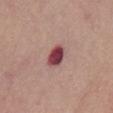Assessment: The lesion was photographed on a routine skin check and not biopsied; there is no pathology result. Acquisition and patient details: This is a white-light tile. The lesion-visualizer software estimated an area of roughly 5.5 mm², an outline eccentricity of about 0.7 (0 = round, 1 = elongated), and a shape-asymmetry score of about 0.15 (0 = symmetric). The analysis additionally found border irregularity of about 1.5 on a 0–10 scale, a within-lesion color-variation index near 4.5/10, and a peripheral color-asymmetry measure near 1.5. The software also gave a classifier nevus-likeness of about 0/100 and a lesion-detection confidence of about 100/100. A female subject aged around 50. On the chest. A close-up tile cropped from a whole-body skin photograph, about 15 mm across.